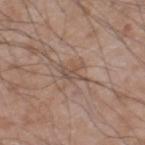Recorded during total-body skin imaging; not selected for excision or biopsy.
On the left thigh.
The patient is a male about 60 years old.
A 15 mm crop from a total-body photograph taken for skin-cancer surveillance.